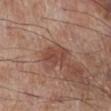Q: Was a biopsy performed?
A: no biopsy performed (imaged during a skin exam)
Q: Who is the patient?
A: male, about 70 years old
Q: Lesion location?
A: the right lower leg
Q: What is the imaging modality?
A: ~15 mm crop, total-body skin-cancer survey